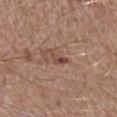Impression:
No biopsy was performed on this lesion — it was imaged during a full skin examination and was not determined to be concerning.
Acquisition and patient details:
A male subject in their mid-60s. The tile uses white-light illumination. The lesion is on the right lower leg. This image is a 15 mm lesion crop taken from a total-body photograph. The lesion's longest dimension is about 3 mm. The lesion-visualizer software estimated lesion-presence confidence of about 100/100.